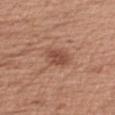follow-up: imaged on a skin check; not biopsied | illumination: white-light | automated lesion analysis: a footprint of about 6 mm², an outline eccentricity of about 0.6 (0 = round, 1 = elongated), and a symmetry-axis asymmetry near 0.2; an average lesion color of about L≈49 a*≈23 b*≈29 (CIELAB); a border-irregularity index near 2/10, internal color variation of about 2.5 on a 0–10 scale, and peripheral color asymmetry of about 1 | acquisition: ~15 mm tile from a whole-body skin photo | lesion diameter: ~3 mm (longest diameter) | subject: female, in their 40s | location: the right upper arm.